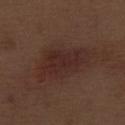Q: Was this lesion biopsied?
A: imaged on a skin check; not biopsied
Q: What is the anatomic site?
A: the right thigh
Q: What is the imaging modality?
A: ~15 mm crop, total-body skin-cancer survey
Q: Who is the patient?
A: male, aged 68–72
Q: What lighting was used for the tile?
A: white-light illumination
Q: How large is the lesion?
A: ~6.5 mm (longest diameter)